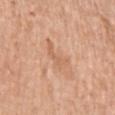The lesion was photographed on a routine skin check and not biopsied; there is no pathology result. A 15 mm close-up tile from a total-body photography series done for melanoma screening. The subject is a female approximately 60 years of age. Located on the arm.